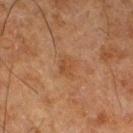Impression: Captured during whole-body skin photography for melanoma surveillance; the lesion was not biopsied. Image and clinical context: A 15 mm close-up tile from a total-body photography series done for melanoma screening. This is a cross-polarized tile. The recorded lesion diameter is about 2.5 mm. Located on the right thigh. A male subject, approximately 80 years of age.